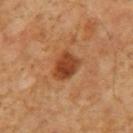notes — no biopsy performed (imaged during a skin exam) | tile lighting — cross-polarized illumination | image source — total-body-photography crop, ~15 mm field of view | automated lesion analysis — a nevus-likeness score of about 95/100 and lesion-presence confidence of about 100/100 | subject — male, roughly 60 years of age | anatomic site — the mid back | size — ≈3.5 mm.